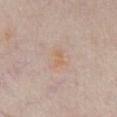Clinical summary: Imaged with white-light lighting. An algorithmic analysis of the crop reported an outline eccentricity of about 0.85 (0 = round, 1 = elongated) and two-axis asymmetry of about 0.35. And it measured a lesion color around L≈63 a*≈17 b*≈33 in CIELAB, roughly 5 lightness units darker than nearby skin, and a normalized lesion–skin contrast near 6.5. And it measured a border-irregularity rating of about 3.5/10, internal color variation of about 0 on a 0–10 scale, and peripheral color asymmetry of about 0. The analysis additionally found an automated nevus-likeness rating near 5 out of 100 and a lesion-detection confidence of about 100/100. A male patient about 60 years old. A lesion tile, about 15 mm wide, cut from a 3D total-body photograph. The lesion is located on the chest.